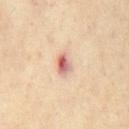The lesion was photographed on a routine skin check and not biopsied; there is no pathology result.
The subject is a male in their 70s.
A lesion tile, about 15 mm wide, cut from a 3D total-body photograph.
The recorded lesion diameter is about 3 mm.
Captured under cross-polarized illumination.
The lesion is on the chest.